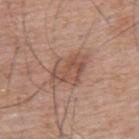| feature | finding |
|---|---|
| biopsy status | catalogued during a skin exam; not biopsied |
| subject | male, approximately 55 years of age |
| size | about 5 mm |
| automated lesion analysis | an area of roughly 10 mm² and a symmetry-axis asymmetry near 0.2; a mean CIELAB color near L≈52 a*≈20 b*≈27; border irregularity of about 3 on a 0–10 scale and radial color variation of about 1.5 |
| illumination | white-light |
| anatomic site | the upper back |
| imaging modality | total-body-photography crop, ~15 mm field of view |